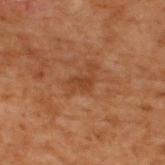No biopsy was performed on this lesion — it was imaged during a full skin examination and was not determined to be concerning. A male subject in their 70s. Cropped from a total-body skin-imaging series; the visible field is about 15 mm. Captured under cross-polarized illumination. The total-body-photography lesion software estimated a lesion color around L≈31 a*≈20 b*≈28 in CIELAB and a lesion-to-skin contrast of about 6 (normalized; higher = more distinct). The lesion is located on the upper back.